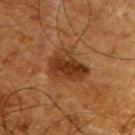The lesion was tiled from a total-body skin photograph and was not biopsied. Imaged with cross-polarized lighting. The lesion-visualizer software estimated a normalized lesion–skin contrast near 9.5. A 15 mm close-up tile from a total-body photography series done for melanoma screening. The lesion's longest dimension is about 5 mm. Located on the upper back. A male patient about 65 years old.